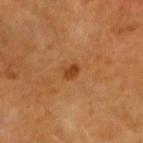workup: catalogued during a skin exam; not biopsied | image: 15 mm crop, total-body photography | lesion size: ~2 mm (longest diameter) | patient: male, aged 58–62 | anatomic site: the head or neck | TBP lesion metrics: a footprint of about 2.5 mm² and a symmetry-axis asymmetry near 0.25; a mean CIELAB color near L≈40 a*≈26 b*≈38 and roughly 9 lightness units darker than nearby skin; a nevus-likeness score of about 95/100 and a detector confidence of about 100 out of 100 that the crop contains a lesion | illumination: cross-polarized illumination.